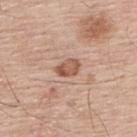Imaged during a routine full-body skin examination; the lesion was not biopsied and no histopathology is available. A male patient about 55 years old. A region of skin cropped from a whole-body photographic capture, roughly 15 mm wide. The lesion is on the back.